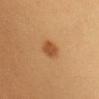Assessment: Captured during whole-body skin photography for melanoma surveillance; the lesion was not biopsied. Image and clinical context: This image is a 15 mm lesion crop taken from a total-body photograph. A female subject approximately 25 years of age. The recorded lesion diameter is about 2.5 mm. The lesion is located on the head or neck. This is a cross-polarized tile.